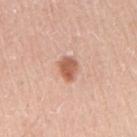Clinical impression: The lesion was tiled from a total-body skin photograph and was not biopsied. Acquisition and patient details: This image is a 15 mm lesion crop taken from a total-body photograph. The recorded lesion diameter is about 2.5 mm. A male subject roughly 60 years of age. Captured under white-light illumination. The lesion is located on the right upper arm.